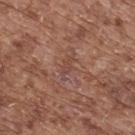This lesion was catalogued during total-body skin photography and was not selected for biopsy. Automated image analysis of the tile measured a lesion color around L≈45 a*≈21 b*≈25 in CIELAB, about 6 CIELAB-L* units darker than the surrounding skin, and a lesion-to-skin contrast of about 5 (normalized; higher = more distinct). And it measured a lesion-detection confidence of about 75/100. Longest diameter approximately 3 mm. The patient is a male aged around 75. On the back. This is a white-light tile. A lesion tile, about 15 mm wide, cut from a 3D total-body photograph.